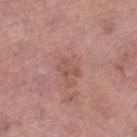notes — imaged on a skin check; not biopsied | patient — female, in their 70s | automated metrics — an area of roughly 4 mm², an outline eccentricity of about 0.8 (0 = round, 1 = elongated), and a symmetry-axis asymmetry near 0.25; about 6 CIELAB-L* units darker than the surrounding skin and a normalized lesion–skin contrast near 5 | body site — the left thigh | image — total-body-photography crop, ~15 mm field of view.